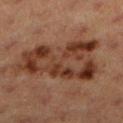Q: Was a biopsy performed?
A: imaged on a skin check; not biopsied
Q: What did automated image analysis measure?
A: a lesion area of about 39 mm², an eccentricity of roughly 0.85, and a symmetry-axis asymmetry near 0.4; a nevus-likeness score of about 0/100 and a detector confidence of about 100 out of 100 that the crop contains a lesion
Q: What kind of image is this?
A: ~15 mm crop, total-body skin-cancer survey
Q: Patient demographics?
A: female, aged 38 to 42
Q: What is the anatomic site?
A: the left lower leg